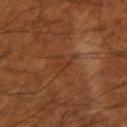| field | value |
|---|---|
| workup | catalogued during a skin exam; not biopsied |
| diameter | about 3 mm |
| image | ~15 mm crop, total-body skin-cancer survey |
| anatomic site | the arm |
| subject | male, aged around 55 |